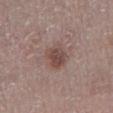Findings:
* workup: catalogued during a skin exam; not biopsied
* body site: the right lower leg
* TBP lesion metrics: a footprint of about 7 mm² and an eccentricity of roughly 0.7; a lesion color around L≈46 a*≈18 b*≈21 in CIELAB, about 10 CIELAB-L* units darker than the surrounding skin, and a normalized lesion–skin contrast near 7.5; a border-irregularity index near 2/10, a color-variation rating of about 3.5/10, and a peripheral color-asymmetry measure near 1
* image source: 15 mm crop, total-body photography
* lighting: white-light illumination
* subject: female, aged approximately 65
* diameter: ~3.5 mm (longest diameter)